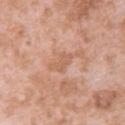lesion diameter: ≈3 mm | illumination: white-light | patient: female, aged 38–42 | image source: 15 mm crop, total-body photography | anatomic site: the upper back | automated metrics: an average lesion color of about L≈60 a*≈22 b*≈33 (CIELAB), a lesion–skin lightness drop of about 7, and a normalized lesion–skin contrast near 5; internal color variation of about 0 on a 0–10 scale and a peripheral color-asymmetry measure near 0.